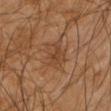biopsy status: total-body-photography surveillance lesion; no biopsy | lesion diameter: ≈5.5 mm | site: the right arm | patient: male, in their 60s | illumination: cross-polarized | automated metrics: a lesion–skin lightness drop of about 6 and a lesion-to-skin contrast of about 5.5 (normalized; higher = more distinct); an automated nevus-likeness rating near 0 out of 100 and a detector confidence of about 95 out of 100 that the crop contains a lesion | image source: total-body-photography crop, ~15 mm field of view.